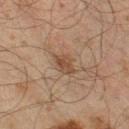Q: Is there a histopathology result?
A: imaged on a skin check; not biopsied
Q: Lesion size?
A: about 3 mm
Q: What is the imaging modality?
A: total-body-photography crop, ~15 mm field of view
Q: Where on the body is the lesion?
A: the right thigh
Q: Illumination type?
A: cross-polarized illumination
Q: Patient demographics?
A: male, aged around 45